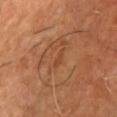Captured during whole-body skin photography for melanoma surveillance; the lesion was not biopsied. This is a cross-polarized tile. Approximately 2.5 mm at its widest. A 15 mm close-up tile from a total-body photography series done for melanoma screening. Located on the front of the torso. A male patient, aged 58 to 62.